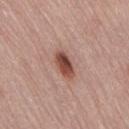<lesion>
<biopsy_status>not biopsied; imaged during a skin examination</biopsy_status>
<patient>
  <sex>female</sex>
  <age_approx>65</age_approx>
</patient>
<site>lower back</site>
<lesion_size>
  <long_diameter_mm_approx>4.0</long_diameter_mm_approx>
</lesion_size>
<automated_metrics>
  <area_mm2_approx>6.0</area_mm2_approx>
  <eccentricity>0.85</eccentricity>
  <shape_asymmetry>0.25</shape_asymmetry>
  <nevus_likeness_0_100>95</nevus_likeness_0_100>
  <lesion_detection_confidence_0_100>100</lesion_detection_confidence_0_100>
</automated_metrics>
<image>
  <source>total-body photography crop</source>
  <field_of_view_mm>15</field_of_view_mm>
</image>
<lighting>white-light</lighting>
</lesion>